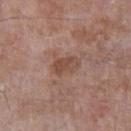No biopsy was performed on this lesion — it was imaged during a full skin examination and was not determined to be concerning. A 15 mm crop from a total-body photograph taken for skin-cancer surveillance. A male subject aged around 65. The lesion is located on the right upper arm. This is a white-light tile. Approximately 3 mm at its widest.